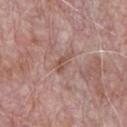Captured during whole-body skin photography for melanoma surveillance; the lesion was not biopsied. The tile uses white-light illumination. Located on the chest. A region of skin cropped from a whole-body photographic capture, roughly 15 mm wide. A male patient, roughly 70 years of age.